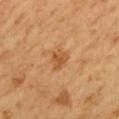Assessment:
This lesion was catalogued during total-body skin photography and was not selected for biopsy.
Image and clinical context:
The lesion is located on the mid back. Automated tile analysis of the lesion measured a border-irregularity index near 3/10 and a within-lesion color-variation index near 2/10. And it measured a classifier nevus-likeness of about 5/100 and a lesion-detection confidence of about 100/100. The subject is a male aged 58 to 62. A 15 mm close-up extracted from a 3D total-body photography capture. This is a cross-polarized tile.